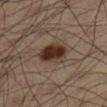<lesion>
<biopsy_status>not biopsied; imaged during a skin examination</biopsy_status>
<lighting>cross-polarized</lighting>
<patient>
  <sex>male</sex>
  <age_approx>35</age_approx>
</patient>
<site>left lower leg</site>
<image>
  <source>total-body photography crop</source>
  <field_of_view_mm>15</field_of_view_mm>
</image>
<automated_metrics>
  <cielab_L>28</cielab_L>
  <cielab_a>15</cielab_a>
  <cielab_b>22</cielab_b>
  <vs_skin_contrast_norm>13.0</vs_skin_contrast_norm>
  <border_irregularity_0_10>1.5</border_irregularity_0_10>
  <color_variation_0_10>4.5</color_variation_0_10>
  <peripheral_color_asymmetry>1.5</peripheral_color_asymmetry>
  <nevus_likeness_0_100>100</nevus_likeness_0_100>
  <lesion_detection_confidence_0_100>100</lesion_detection_confidence_0_100>
</automated_metrics>
</lesion>